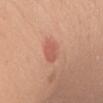Q: Is there a histopathology result?
A: total-body-photography surveillance lesion; no biopsy
Q: What did automated image analysis measure?
A: border irregularity of about 3 on a 0–10 scale and internal color variation of about 2.5 on a 0–10 scale; an automated nevus-likeness rating near 45 out of 100
Q: How was the tile lit?
A: white-light illumination
Q: What is the lesion's diameter?
A: ≈4 mm
Q: What kind of image is this?
A: ~15 mm crop, total-body skin-cancer survey
Q: Who is the patient?
A: female, about 55 years old
Q: Lesion location?
A: the chest